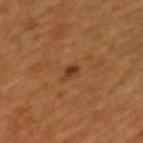notes: no biopsy performed (imaged during a skin exam) | body site: the mid back | automated lesion analysis: a shape eccentricity near 0.75 and a shape-asymmetry score of about 0.35 (0 = symmetric); a lesion color around L≈34 a*≈22 b*≈33 in CIELAB, roughly 9 lightness units darker than nearby skin, and a lesion-to-skin contrast of about 8.5 (normalized; higher = more distinct); a border-irregularity index near 3/10, internal color variation of about 0 on a 0–10 scale, and radial color variation of about 0; a nevus-likeness score of about 90/100 and lesion-presence confidence of about 100/100 | illumination: cross-polarized illumination | lesion diameter: ≈2 mm | image source: total-body-photography crop, ~15 mm field of view | subject: male, aged 43–47.